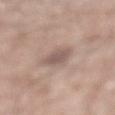Clinical summary: A close-up tile cropped from a whole-body skin photograph, about 15 mm across. Automated image analysis of the tile measured an outline eccentricity of about 0.8 (0 = round, 1 = elongated) and a symmetry-axis asymmetry near 0.2. It also reported a classifier nevus-likeness of about 0/100 and a lesion-detection confidence of about 100/100. Captured under white-light illumination. A male patient, aged 58–62. From the mid back. About 3.5 mm across.